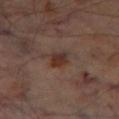<lesion>
  <biopsy_status>not biopsied; imaged during a skin examination</biopsy_status>
  <image>
    <source>total-body photography crop</source>
    <field_of_view_mm>15</field_of_view_mm>
  </image>
  <patient>
    <sex>male</sex>
    <age_approx>70</age_approx>
  </patient>
  <site>leg</site>
  <lighting>cross-polarized</lighting>
  <lesion_size>
    <long_diameter_mm_approx>2.5</long_diameter_mm_approx>
  </lesion_size>
</lesion>